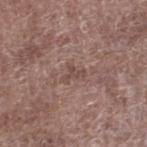{
  "biopsy_status": "not biopsied; imaged during a skin examination",
  "lighting": "white-light",
  "site": "right lower leg",
  "image": {
    "source": "total-body photography crop",
    "field_of_view_mm": 15
  },
  "patient": {
    "sex": "male",
    "age_approx": 70
  },
  "lesion_size": {
    "long_diameter_mm_approx": 2.5
  }
}